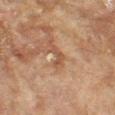{
  "biopsy_status": "not biopsied; imaged during a skin examination",
  "automated_metrics": {
    "border_irregularity_0_10": 6.5,
    "color_variation_0_10": 0.0,
    "peripheral_color_asymmetry": 0.0,
    "nevus_likeness_0_100": 0,
    "lesion_detection_confidence_0_100": 80
  },
  "lighting": "cross-polarized",
  "patient": {
    "sex": "female",
    "age_approx": 80
  },
  "image": {
    "source": "total-body photography crop",
    "field_of_view_mm": 15
  },
  "site": "left forearm"
}